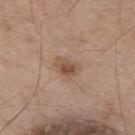Context:
Captured under white-light illumination. Located on the upper back. The patient is a male about 70 years old. Longest diameter approximately 3 mm. A lesion tile, about 15 mm wide, cut from a 3D total-body photograph. Automated image analysis of the tile measured a lesion area of about 5.5 mm², a shape eccentricity near 0.8, and a shape-asymmetry score of about 0.25 (0 = symmetric). It also reported a mean CIELAB color near L≈51 a*≈18 b*≈29 and a normalized lesion–skin contrast near 7.